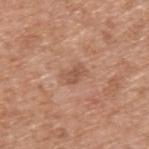Captured during whole-body skin photography for melanoma surveillance; the lesion was not biopsied. The patient is a male aged approximately 65. On the back. Imaged with white-light lighting. This image is a 15 mm lesion crop taken from a total-body photograph. Approximately 3 mm at its widest.